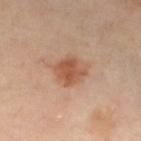The lesion was photographed on a routine skin check and not biopsied; there is no pathology result.